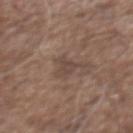Impression:
The lesion was photographed on a routine skin check and not biopsied; there is no pathology result.
Image and clinical context:
The lesion is on the chest. A 15 mm close-up extracted from a 3D total-body photography capture. A male patient, roughly 75 years of age.